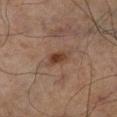A 15 mm crop from a total-body photograph taken for skin-cancer surveillance.
Located on the right lower leg.
Approximately 2.5 mm at its widest.
A male patient approximately 65 years of age.
The total-body-photography lesion software estimated a footprint of about 3.5 mm² and a shape-asymmetry score of about 0.3 (0 = symmetric). The analysis additionally found a lesion color around L≈30 a*≈16 b*≈23 in CIELAB, a lesion–skin lightness drop of about 8, and a normalized lesion–skin contrast near 9. The analysis additionally found a border-irregularity rating of about 3/10, a within-lesion color-variation index near 3/10, and radial color variation of about 1.